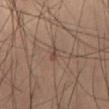Impression:
No biopsy was performed on this lesion — it was imaged during a full skin examination and was not determined to be concerning.
Background:
On the right thigh. The total-body-photography lesion software estimated an average lesion color of about L≈43 a*≈16 b*≈23 (CIELAB) and a lesion-to-skin contrast of about 5.5 (normalized; higher = more distinct). The software also gave internal color variation of about 0 on a 0–10 scale and a peripheral color-asymmetry measure near 0. A 15 mm crop from a total-body photograph taken for skin-cancer surveillance. This is a cross-polarized tile. A male patient, about 60 years old. Measured at roughly 2.5 mm in maximum diameter.